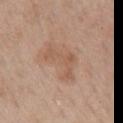Clinical impression: The lesion was photographed on a routine skin check and not biopsied; there is no pathology result. Clinical summary: Located on the front of the torso. A 15 mm crop from a total-body photograph taken for skin-cancer surveillance. This is a white-light tile. A female patient in their mid- to late 50s.